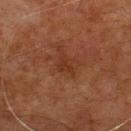A roughly 15 mm field-of-view crop from a total-body skin photograph. Longest diameter approximately 3 mm. The tile uses cross-polarized illumination. The lesion is located on the back. A male subject, aged around 80.